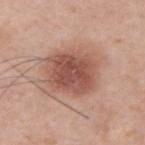Clinical impression:
Imaged during a routine full-body skin examination; the lesion was not biopsied and no histopathology is available.
Clinical summary:
The lesion is on the upper back. A male patient in their mid- to late 30s. Captured under white-light illumination. About 5.5 mm across. Automated tile analysis of the lesion measured a footprint of about 21 mm², an eccentricity of roughly 0.5, and two-axis asymmetry of about 0.15. The software also gave a mean CIELAB color near L≈52 a*≈23 b*≈27, about 13 CIELAB-L* units darker than the surrounding skin, and a lesion-to-skin contrast of about 9 (normalized; higher = more distinct). A 15 mm crop from a total-body photograph taken for skin-cancer surveillance.